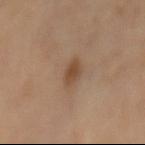No biopsy was performed on this lesion — it was imaged during a full skin examination and was not determined to be concerning.
Located on the mid back.
A 15 mm close-up extracted from a 3D total-body photography capture.